Cropped from a whole-body photographic skin survey; the tile spans about 15 mm; on the mid back; a male subject, in their 70s:
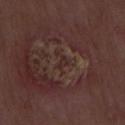Imaged with white-light lighting.
The recorded lesion diameter is about 1.5 mm.
Histopathological examination showed a squamous cell carcinoma in situ.